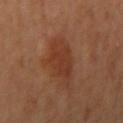No biopsy was performed on this lesion — it was imaged during a full skin examination and was not determined to be concerning. The subject is a female aged approximately 65. On the left arm. This image is a 15 mm lesion crop taken from a total-body photograph.